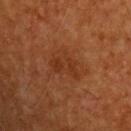Assessment:
Part of a total-body skin-imaging series; this lesion was reviewed on a skin check and was not flagged for biopsy.
Clinical summary:
Located on the upper back. A male subject, aged around 60. Approximately 4.5 mm at its widest. A 15 mm close-up tile from a total-body photography series done for melanoma screening.